An algorithmic analysis of the crop reported a footprint of about 28 mm², a shape eccentricity near 0.5, and a symmetry-axis asymmetry near 0.15. It also reported a border-irregularity index near 1.5/10, internal color variation of about 8.5 on a 0–10 scale, and radial color variation of about 2.5. The software also gave a classifier nevus-likeness of about 100/100 and a lesion-detection confidence of about 100/100 · a male subject, approximately 45 years of age · located on the left upper arm · a region of skin cropped from a whole-body photographic capture, roughly 15 mm wide · the tile uses cross-polarized illumination.
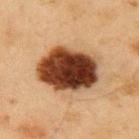The lesion was biopsied, and histopathology showed a melanoma in situ (malignant).